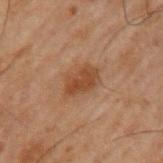Assessment:
The lesion was tiled from a total-body skin photograph and was not biopsied.
Clinical summary:
A male subject, aged 58–62. A roughly 15 mm field-of-view crop from a total-body skin photograph. On the left upper arm. Approximately 4 mm at its widest.